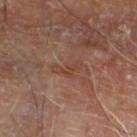Captured under cross-polarized illumination. A male patient in their 70s. From the leg. The total-body-photography lesion software estimated roughly 6 lightness units darker than nearby skin. The analysis additionally found a border-irregularity index near 6.5/10, internal color variation of about 0 on a 0–10 scale, and a peripheral color-asymmetry measure near 0. A 15 mm close-up tile from a total-body photography series done for melanoma screening.